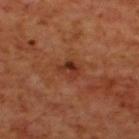On the upper back. Measured at roughly 2.5 mm in maximum diameter. Cropped from a whole-body photographic skin survey; the tile spans about 15 mm. A male patient, roughly 70 years of age. The tile uses cross-polarized illumination.